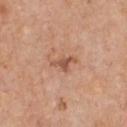Assessment: Part of a total-body skin-imaging series; this lesion was reviewed on a skin check and was not flagged for biopsy. Clinical summary: Automated image analysis of the tile measured an average lesion color of about L≈54 a*≈23 b*≈31 (CIELAB), about 11 CIELAB-L* units darker than the surrounding skin, and a normalized lesion–skin contrast near 7.5. Measured at roughly 3 mm in maximum diameter. Cropped from a total-body skin-imaging series; the visible field is about 15 mm. The tile uses white-light illumination. A male patient, aged 58 to 62. The lesion is located on the chest.